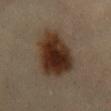The lesion was tiled from a total-body skin photograph and was not biopsied. The lesion is on the back. Measured at roughly 7.5 mm in maximum diameter. A female subject aged approximately 45. Cropped from a total-body skin-imaging series; the visible field is about 15 mm. The tile uses cross-polarized illumination.